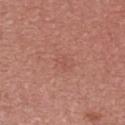Notes:
* biopsy status — total-body-photography surveillance lesion; no biopsy
* image — ~15 mm tile from a whole-body skin photo
* subject — male, in their 40s
* lesion diameter — ≈1.5 mm
* body site — the upper back
* illumination — white-light